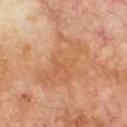Image and clinical context:
From the front of the torso. A male subject roughly 70 years of age. A 15 mm crop from a total-body photograph taken for skin-cancer surveillance.